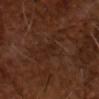Recorded during total-body skin imaging; not selected for excision or biopsy. A male subject, in their mid- to late 60s. A 15 mm close-up tile from a total-body photography series done for melanoma screening.